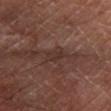Acquisition and patient details: An algorithmic analysis of the crop reported a lesion area of about 3 mm², a shape eccentricity near 0.85, and a symmetry-axis asymmetry near 0.3. The analysis additionally found an average lesion color of about L≈29 a*≈17 b*≈20 (CIELAB) and a lesion-to-skin contrast of about 5 (normalized; higher = more distinct). It also reported radial color variation of about 0.5. The analysis additionally found an automated nevus-likeness rating near 0 out of 100 and a lesion-detection confidence of about 60/100. A male patient approximately 65 years of age. The lesion is located on the left lower leg. The tile uses cross-polarized illumination. About 2.5 mm across. A 15 mm crop from a total-body photograph taken for skin-cancer surveillance.